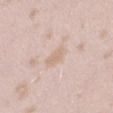This lesion was catalogued during total-body skin photography and was not selected for biopsy.